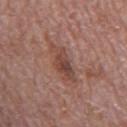Assessment:
This lesion was catalogued during total-body skin photography and was not selected for biopsy.
Clinical summary:
Measured at roughly 5 mm in maximum diameter. The lesion is located on the mid back. The patient is a male aged approximately 70. A 15 mm crop from a total-body photograph taken for skin-cancer surveillance. Captured under white-light illumination.